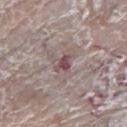| field | value |
|---|---|
| anatomic site | the leg |
| automated lesion analysis | roughly 11 lightness units darker than nearby skin and a normalized lesion–skin contrast near 8.5; border irregularity of about 4 on a 0–10 scale and a color-variation rating of about 1.5/10 |
| illumination | white-light |
| patient | female, roughly 85 years of age or older |
| size | ≈2.5 mm |
| acquisition | 15 mm crop, total-body photography |